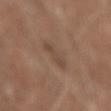This lesion was catalogued during total-body skin photography and was not selected for biopsy.
The lesion is located on the arm.
The subject is a male aged 58 to 62.
A roughly 15 mm field-of-view crop from a total-body skin photograph.
Measured at roughly 3 mm in maximum diameter.